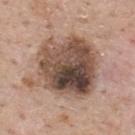Case summary:
* follow-up: catalogued during a skin exam; not biopsied
* lesion size: ~8 mm (longest diameter)
* TBP lesion metrics: a lesion color around L≈49 a*≈16 b*≈25 in CIELAB, a lesion–skin lightness drop of about 17, and a normalized border contrast of about 11.5; a border-irregularity index near 3/10 and a within-lesion color-variation index near 10/10; a nevus-likeness score of about 10/100
* image: 15 mm crop, total-body photography
* subject: male, aged 58–62
* body site: the upper back
* tile lighting: white-light illumination